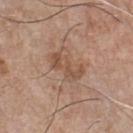This lesion was catalogued during total-body skin photography and was not selected for biopsy. Imaged with white-light lighting. A male subject aged approximately 75. The lesion's longest dimension is about 4.5 mm. A 15 mm close-up extracted from a 3D total-body photography capture. From the chest.